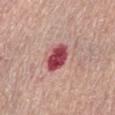workup = total-body-photography surveillance lesion; no biopsy
diameter = ~4 mm (longest diameter)
lighting = white-light
subject = female, in their mid- to late 60s
site = the abdomen
image = ~15 mm tile from a whole-body skin photo